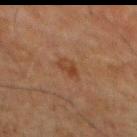  biopsy_status: not biopsied; imaged during a skin examination
  patient:
    sex: male
    age_approx: 80
  lighting: cross-polarized
  image:
    source: total-body photography crop
    field_of_view_mm: 15
  site: abdomen